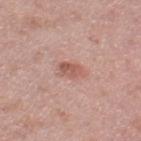Assessment:
This lesion was catalogued during total-body skin photography and was not selected for biopsy.
Context:
This image is a 15 mm lesion crop taken from a total-body photograph. Automated image analysis of the tile measured an average lesion color of about L≈56 a*≈24 b*≈27 (CIELAB), roughly 10 lightness units darker than nearby skin, and a lesion-to-skin contrast of about 6.5 (normalized; higher = more distinct). Captured under white-light illumination. Approximately 3 mm at its widest. A female patient, roughly 40 years of age. The lesion is located on the right thigh.